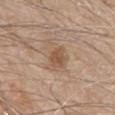Assessment: This lesion was catalogued during total-body skin photography and was not selected for biopsy.